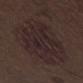Recorded during total-body skin imaging; not selected for excision or biopsy. The lesion is on the leg. Measured at roughly 10.5 mm in maximum diameter. Automated image analysis of the tile measured a lesion–skin lightness drop of about 5 and a normalized lesion–skin contrast near 6.5. A 15 mm close-up tile from a total-body photography series done for melanoma screening. The patient is a male aged 68–72.